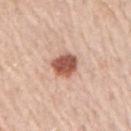Q: Was a biopsy performed?
A: imaged on a skin check; not biopsied
Q: Lesion size?
A: about 3 mm
Q: Lesion location?
A: the right upper arm
Q: What are the patient's age and sex?
A: male, aged 63–67
Q: What is the imaging modality?
A: 15 mm crop, total-body photography
Q: What lighting was used for the tile?
A: white-light